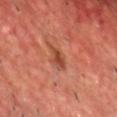Q: Is there a histopathology result?
A: imaged on a skin check; not biopsied
Q: Where on the body is the lesion?
A: the front of the torso
Q: What are the patient's age and sex?
A: male, roughly 50 years of age
Q: How was this image acquired?
A: ~15 mm crop, total-body skin-cancer survey
Q: Illumination type?
A: cross-polarized illumination